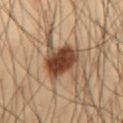Q: Was this lesion biopsied?
A: no biopsy performed (imaged during a skin exam)
Q: Where on the body is the lesion?
A: the abdomen
Q: Automated lesion metrics?
A: an average lesion color of about L≈36 a*≈16 b*≈26 (CIELAB), a lesion–skin lightness drop of about 13, and a normalized border contrast of about 11.5; a border-irregularity rating of about 4/10, internal color variation of about 5.5 on a 0–10 scale, and peripheral color asymmetry of about 1.5
Q: What is the imaging modality?
A: ~15 mm tile from a whole-body skin photo
Q: Patient demographics?
A: male, in their mid- to late 50s
Q: Lesion size?
A: ≈5 mm